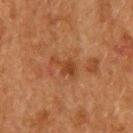The lesion-visualizer software estimated a normalized border contrast of about 6.5.
The lesion is located on the upper back.
Captured under cross-polarized illumination.
Cropped from a total-body skin-imaging series; the visible field is about 15 mm.
The lesion's longest dimension is about 3.5 mm.
A male subject about 50 years old.